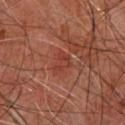lesion size = ~3 mm (longest diameter)
illumination = cross-polarized
image source = 15 mm crop, total-body photography
body site = the upper back
patient = male, roughly 60 years of age